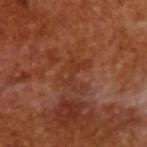{"biopsy_status": "not biopsied; imaged during a skin examination", "lighting": "cross-polarized", "lesion_size": {"long_diameter_mm_approx": 4.0}, "image": {"source": "total-body photography crop", "field_of_view_mm": 15}, "patient": {"sex": "male", "age_approx": 65}}